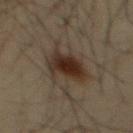Impression: The lesion was photographed on a routine skin check and not biopsied; there is no pathology result. Background: On the mid back. A roughly 15 mm field-of-view crop from a total-body skin photograph. A male subject, aged around 35.